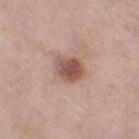Part of a total-body skin-imaging series; this lesion was reviewed on a skin check and was not flagged for biopsy. Imaged with white-light lighting. From the right thigh. A female patient, approximately 55 years of age. A 15 mm close-up extracted from a 3D total-body photography capture. About 3.5 mm across.